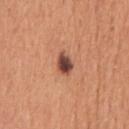Assessment: The lesion was tiled from a total-body skin photograph and was not biopsied. Acquisition and patient details: A roughly 15 mm field-of-view crop from a total-body skin photograph. The lesion is located on the chest. Measured at roughly 3 mm in maximum diameter. A male subject aged 53 to 57. Captured under white-light illumination.